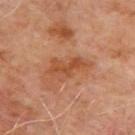Q: Was a biopsy performed?
A: catalogued during a skin exam; not biopsied
Q: Patient demographics?
A: male, aged around 65
Q: What kind of image is this?
A: 15 mm crop, total-body photography
Q: Where on the body is the lesion?
A: the upper back